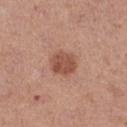{"biopsy_status": "not biopsied; imaged during a skin examination", "patient": {"sex": "female", "age_approx": 40}, "automated_metrics": {"cielab_L": 51, "cielab_a": 24, "cielab_b": 29, "vs_skin_darker_L": 11.0, "vs_skin_contrast_norm": 8.0, "color_variation_0_10": 3.0, "peripheral_color_asymmetry": 1.0}, "image": {"source": "total-body photography crop", "field_of_view_mm": 15}, "site": "left thigh"}